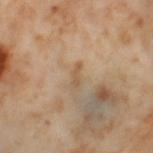Imaged during a routine full-body skin examination; the lesion was not biopsied and no histopathology is available.
On the left thigh.
A region of skin cropped from a whole-body photographic capture, roughly 15 mm wide.
A female subject in their mid- to late 50s.
Approximately 2.5 mm at its widest.